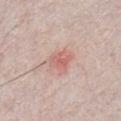biopsy status: total-body-photography surveillance lesion; no biopsy
image source: 15 mm crop, total-body photography
tile lighting: white-light illumination
image-analysis metrics: a color-variation rating of about 3/10 and radial color variation of about 1; an automated nevus-likeness rating near 20 out of 100 and a detector confidence of about 100 out of 100 that the crop contains a lesion
subject: male, in their mid-20s
location: the chest